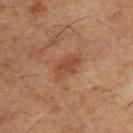The total-body-photography lesion software estimated a shape-asymmetry score of about 0.25 (0 = symmetric). And it measured about 8 CIELAB-L* units darker than the surrounding skin and a normalized border contrast of about 6.5. The software also gave a nevus-likeness score of about 20/100 and a lesion-detection confidence of about 100/100. Measured at roughly 4 mm in maximum diameter. Imaged with cross-polarized lighting. The lesion is located on the upper back. A close-up tile cropped from a whole-body skin photograph, about 15 mm across. A male subject, aged 53 to 57.